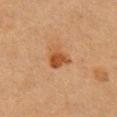Q: Was this lesion biopsied?
A: total-body-photography surveillance lesion; no biopsy
Q: Lesion size?
A: about 3 mm
Q: What lighting was used for the tile?
A: cross-polarized
Q: What are the patient's age and sex?
A: female, aged approximately 40
Q: What is the anatomic site?
A: the right upper arm
Q: How was this image acquired?
A: ~15 mm tile from a whole-body skin photo